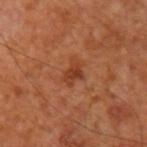Assessment: Part of a total-body skin-imaging series; this lesion was reviewed on a skin check and was not flagged for biopsy. Image and clinical context: The total-body-photography lesion software estimated a lesion area of about 4.5 mm². The analysis additionally found an average lesion color of about L≈40 a*≈28 b*≈35 (CIELAB), a lesion–skin lightness drop of about 8, and a lesion-to-skin contrast of about 7 (normalized; higher = more distinct). The software also gave border irregularity of about 3.5 on a 0–10 scale and peripheral color asymmetry of about 1. A 15 mm close-up tile from a total-body photography series done for melanoma screening. About 3 mm across. Imaged with cross-polarized lighting. The patient is about 65 years old. From the left upper arm.